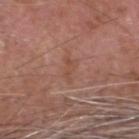Captured during whole-body skin photography for melanoma surveillance; the lesion was not biopsied.
The patient is a male approximately 75 years of age.
Longest diameter approximately 3 mm.
Located on the head or neck.
Cropped from a whole-body photographic skin survey; the tile spans about 15 mm.